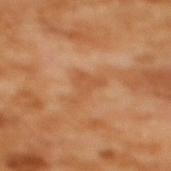Impression:
Imaged during a routine full-body skin examination; the lesion was not biopsied and no histopathology is available.
Image and clinical context:
Located on the back. The recorded lesion diameter is about 4.5 mm. A 15 mm close-up tile from a total-body photography series done for melanoma screening. A female subject, approximately 55 years of age. This is a cross-polarized tile.